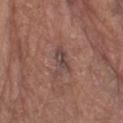follow-up: total-body-photography surveillance lesion; no biopsy